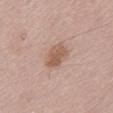Q: Was this lesion biopsied?
A: imaged on a skin check; not biopsied
Q: Lesion size?
A: ~3.5 mm (longest diameter)
Q: What are the patient's age and sex?
A: male, about 50 years old
Q: What kind of image is this?
A: ~15 mm crop, total-body skin-cancer survey
Q: What is the anatomic site?
A: the lower back
Q: Illumination type?
A: white-light